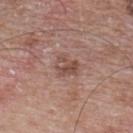Findings:
* follow-up — no biopsy performed (imaged during a skin exam)
* diameter — ≈2.5 mm
* body site — the upper back
* imaging modality — ~15 mm tile from a whole-body skin photo
* subject — male, in their mid-60s
* illumination — white-light